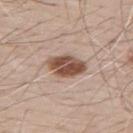{"site": "back", "image": {"source": "total-body photography crop", "field_of_view_mm": 15}, "patient": {"sex": "male", "age_approx": 70}, "lesion_size": {"long_diameter_mm_approx": 5.0}}